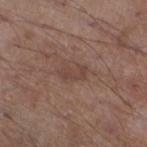{
  "biopsy_status": "not biopsied; imaged during a skin examination",
  "patient": {
    "sex": "male",
    "age_approx": 55
  },
  "image": {
    "source": "total-body photography crop",
    "field_of_view_mm": 15
  },
  "site": "left lower leg",
  "lesion_size": {
    "long_diameter_mm_approx": 3.0
  },
  "automated_metrics": {
    "border_irregularity_0_10": 5.0,
    "color_variation_0_10": 0.5,
    "peripheral_color_asymmetry": 0.0
  }
}